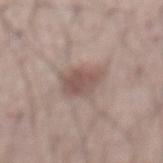Clinical impression:
Imaged during a routine full-body skin examination; the lesion was not biopsied and no histopathology is available.
Image and clinical context:
This is a white-light tile. An algorithmic analysis of the crop reported an area of roughly 9.5 mm², an outline eccentricity of about 0.55 (0 = round, 1 = elongated), and a shape-asymmetry score of about 0.2 (0 = symmetric). It also reported an automated nevus-likeness rating near 85 out of 100 and a lesion-detection confidence of about 100/100. From the abdomen. A region of skin cropped from a whole-body photographic capture, roughly 15 mm wide. A male subject approximately 55 years of age. Longest diameter approximately 4 mm.